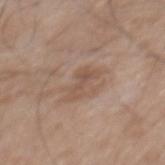follow-up = catalogued during a skin exam; not biopsied | lesion diameter = about 4 mm | patient = male, aged 73 to 77 | imaging modality = ~15 mm crop, total-body skin-cancer survey | body site = the mid back | illumination = white-light illumination.